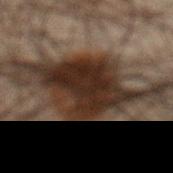biopsy status=imaged on a skin check; not biopsied
location=the abdomen
imaging modality=15 mm crop, total-body photography
diameter=≈8.5 mm
TBP lesion metrics=about 15 CIELAB-L* units darker than the surrounding skin and a normalized lesion–skin contrast near 15.5; a nevus-likeness score of about 100/100 and a lesion-detection confidence of about 95/100
patient=male, aged 63–67